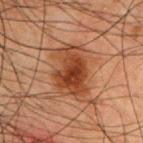Impression:
This lesion was catalogued during total-body skin photography and was not selected for biopsy.
Acquisition and patient details:
A male subject, aged 48 to 52. Longest diameter approximately 5.5 mm. Imaged with cross-polarized lighting. The lesion is located on the chest. A lesion tile, about 15 mm wide, cut from a 3D total-body photograph.